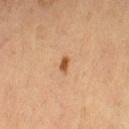  image:
    source: total-body photography crop
    field_of_view_mm: 15
  site: leg
  patient:
    sex: female
    age_approx: 70
  lighting: cross-polarized
  lesion_size:
    long_diameter_mm_approx: 2.0